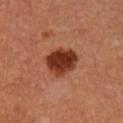The lesion was photographed on a routine skin check and not biopsied; there is no pathology result. Automated tile analysis of the lesion measured an area of roughly 12 mm², a shape eccentricity near 0.35, and a shape-asymmetry score of about 0.2 (0 = symmetric). The software also gave a nevus-likeness score of about 100/100 and lesion-presence confidence of about 100/100. The tile uses cross-polarized illumination. Cropped from a whole-body photographic skin survey; the tile spans about 15 mm. The recorded lesion diameter is about 4 mm. The lesion is on the right forearm. A female subject approximately 35 years of age.